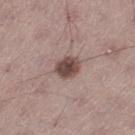biopsy_status: not biopsied; imaged during a skin examination
lesion_size:
  long_diameter_mm_approx: 3.5
patient:
  sex: male
  age_approx: 45
site: left thigh
lighting: white-light
image:
  source: total-body photography crop
  field_of_view_mm: 15